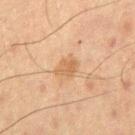Recorded during total-body skin imaging; not selected for excision or biopsy.
The tile uses cross-polarized illumination.
An algorithmic analysis of the crop reported a lesion area of about 4.5 mm², a shape eccentricity near 0.7, and a symmetry-axis asymmetry near 0.25. The analysis additionally found a classifier nevus-likeness of about 0/100 and a lesion-detection confidence of about 100/100.
The patient is a male roughly 60 years of age.
A roughly 15 mm field-of-view crop from a total-body skin photograph.
Measured at roughly 3 mm in maximum diameter.